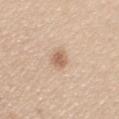Q: Automated lesion metrics?
A: border irregularity of about 3 on a 0–10 scale
Q: Patient demographics?
A: female, roughly 25 years of age
Q: Illumination type?
A: white-light
Q: Where on the body is the lesion?
A: the left upper arm
Q: Lesion size?
A: about 3 mm
Q: What is the imaging modality?
A: total-body-photography crop, ~15 mm field of view